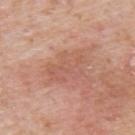Background: On the upper back. A male patient, aged 73–77. A 15 mm crop from a total-body photograph taken for skin-cancer surveillance.